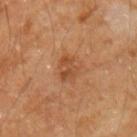<lesion>
  <lesion_size>
    <long_diameter_mm_approx>3.5</long_diameter_mm_approx>
  </lesion_size>
  <lighting>cross-polarized</lighting>
  <image>
    <source>total-body photography crop</source>
    <field_of_view_mm>15</field_of_view_mm>
  </image>
  <automated_metrics>
    <vs_skin_contrast_norm>5.5</vs_skin_contrast_norm>
    <border_irregularity_0_10>2.5</border_irregularity_0_10>
    <peripheral_color_asymmetry>1.5</peripheral_color_asymmetry>
    <nevus_likeness_0_100>10</nevus_likeness_0_100>
    <lesion_detection_confidence_0_100>100</lesion_detection_confidence_0_100>
  </automated_metrics>
  <site>left upper arm</site>
  <patient>
    <sex>male</sex>
    <age_approx>60</age_approx>
  </patient>
</lesion>